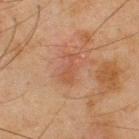| field | value |
|---|---|
| biopsy status | total-body-photography surveillance lesion; no biopsy |
| imaging modality | ~15 mm tile from a whole-body skin photo |
| automated metrics | an area of roughly 5 mm², an eccentricity of roughly 0.9, and a shape-asymmetry score of about 0.45 (0 = symmetric); a lesion color around L≈42 a*≈20 b*≈28 in CIELAB, roughly 5 lightness units darker than nearby skin, and a normalized lesion–skin contrast near 4.5; border irregularity of about 5.5 on a 0–10 scale, a within-lesion color-variation index near 1/10, and radial color variation of about 0; a lesion-detection confidence of about 100/100 |
| location | the back |
| patient | male, in their mid- to late 40s |
| illumination | cross-polarized |